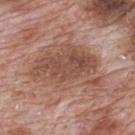<tbp_lesion>
<biopsy_status>not biopsied; imaged during a skin examination</biopsy_status>
<patient>
  <sex>male</sex>
  <age_approx>70</age_approx>
</patient>
<site>mid back</site>
<lighting>white-light</lighting>
<image>
  <source>total-body photography crop</source>
  <field_of_view_mm>15</field_of_view_mm>
</image>
<lesion_size>
  <long_diameter_mm_approx>8.5</long_diameter_mm_approx>
</lesion_size>
<automated_metrics>
  <area_mm2_approx>35.0</area_mm2_approx>
  <eccentricity>0.75</eccentricity>
  <cielab_L>51</cielab_L>
  <cielab_a>21</cielab_a>
  <cielab_b>27</cielab_b>
  <vs_skin_darker_L>9.0</vs_skin_darker_L>
  <vs_skin_contrast_norm>6.5</vs_skin_contrast_norm>
  <border_irregularity_0_10>4.0</border_irregularity_0_10>
  <color_variation_0_10>5.0</color_variation_0_10>
  <peripheral_color_asymmetry>2.0</peripheral_color_asymmetry>
  <nevus_likeness_0_100>0</nevus_likeness_0_100>
  <lesion_detection_confidence_0_100>75</lesion_detection_confidence_0_100>
</automated_metrics>
</tbp_lesion>